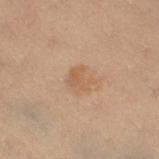Acquisition and patient details: About 2.5 mm across. Imaged with cross-polarized lighting. On the right thigh. This image is a 15 mm lesion crop taken from a total-body photograph. A female subject approximately 40 years of age. The total-body-photography lesion software estimated an area of roughly 4.5 mm², an eccentricity of roughly 0.4, and a symmetry-axis asymmetry near 0.35. The software also gave about 6 CIELAB-L* units darker than the surrounding skin and a normalized border contrast of about 5.5.